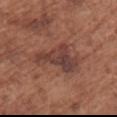{"biopsy_status": "not biopsied; imaged during a skin examination", "image": {"source": "total-body photography crop", "field_of_view_mm": 15}, "automated_metrics": {"area_mm2_approx": 15.0, "eccentricity": 0.85, "cielab_L": 41, "cielab_a": 22, "cielab_b": 24, "vs_skin_darker_L": 9.0, "vs_skin_contrast_norm": 8.0, "border_irregularity_0_10": 6.0, "color_variation_0_10": 5.5, "nevus_likeness_0_100": 0, "lesion_detection_confidence_0_100": 100}, "patient": {"sex": "female", "age_approx": 75}, "lighting": "white-light", "site": "upper back", "lesion_size": {"long_diameter_mm_approx": 6.5}}